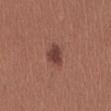<case>
  <biopsy_status>not biopsied; imaged during a skin examination</biopsy_status>
  <site>abdomen</site>
  <image>
    <source>total-body photography crop</source>
    <field_of_view_mm>15</field_of_view_mm>
  </image>
  <patient>
    <sex>female</sex>
    <age_approx>25</age_approx>
  </patient>
  <lighting>white-light</lighting>
  <lesion_size>
    <long_diameter_mm_approx>3.0</long_diameter_mm_approx>
  </lesion_size>
</case>